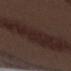| key | value |
|---|---|
| workup | catalogued during a skin exam; not biopsied |